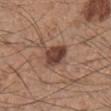Findings:
- image source: ~15 mm tile from a whole-body skin photo
- patient: male, aged 33–37
- anatomic site: the leg